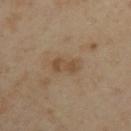This lesion was catalogued during total-body skin photography and was not selected for biopsy.
A female subject, roughly 40 years of age.
A close-up tile cropped from a whole-body skin photograph, about 15 mm across.
The lesion is located on the left upper arm.
About 3 mm across.